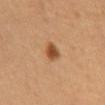{
  "biopsy_status": "not biopsied; imaged during a skin examination",
  "automated_metrics": {
    "border_irregularity_0_10": 2.0,
    "peripheral_color_asymmetry": 1.0,
    "nevus_likeness_0_100": 100,
    "lesion_detection_confidence_0_100": 100
  },
  "patient": {
    "sex": "male",
    "age_approx": 60
  },
  "image": {
    "source": "total-body photography crop",
    "field_of_view_mm": 15
  },
  "lighting": "cross-polarized",
  "site": "mid back"
}